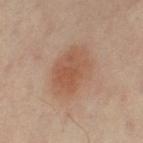Notes:
- acquisition — total-body-photography crop, ~15 mm field of view
- location — the left thigh
- patient — female, aged 38 to 42